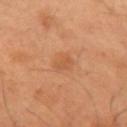Assessment: Recorded during total-body skin imaging; not selected for excision or biopsy. Image and clinical context: A close-up tile cropped from a whole-body skin photograph, about 15 mm across. The lesion is on the arm. A male patient, aged 48–52. The tile uses cross-polarized illumination. An algorithmic analysis of the crop reported a lesion area of about 4.5 mm², an eccentricity of roughly 0.55, and two-axis asymmetry of about 0.35. The software also gave a mean CIELAB color near L≈55 a*≈24 b*≈37 and a normalized lesion–skin contrast near 4.5. And it measured a within-lesion color-variation index near 2/10 and radial color variation of about 1. Measured at roughly 2.5 mm in maximum diameter.